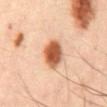  biopsy_status: not biopsied; imaged during a skin examination
  lighting: cross-polarized
  image:
    source: total-body photography crop
    field_of_view_mm: 15
  automated_metrics:
    cielab_L: 58
    cielab_a: 26
    cielab_b: 37
    vs_skin_darker_L: 19.0
    vs_skin_contrast_norm: 11.5
    border_irregularity_0_10: 1.5
    color_variation_0_10: 7.0
    nevus_likeness_0_100: 100
    lesion_detection_confidence_0_100: 100
  site: mid back
  patient:
    sex: male
    age_approx: 50
  lesion_size:
    long_diameter_mm_approx: 4.0